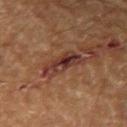notes: catalogued during a skin exam; not biopsied
imaging modality: ~15 mm crop, total-body skin-cancer survey
illumination: cross-polarized
lesion size: ~4.5 mm (longest diameter)
subject: male, aged approximately 60
automated metrics: a mean CIELAB color near L≈30 a*≈20 b*≈22, about 9 CIELAB-L* units darker than the surrounding skin, and a lesion-to-skin contrast of about 10 (normalized; higher = more distinct); border irregularity of about 3.5 on a 0–10 scale, internal color variation of about 8.5 on a 0–10 scale, and radial color variation of about 3; a classifier nevus-likeness of about 0/100 and lesion-presence confidence of about 100/100